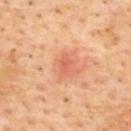Part of a total-body skin-imaging series; this lesion was reviewed on a skin check and was not flagged for biopsy.
Located on the upper back.
Automated image analysis of the tile measured a mean CIELAB color near L≈62 a*≈33 b*≈36, about 8 CIELAB-L* units darker than the surrounding skin, and a normalized lesion–skin contrast near 5. And it measured border irregularity of about 2.5 on a 0–10 scale, internal color variation of about 0 on a 0–10 scale, and radial color variation of about 0. It also reported a nevus-likeness score of about 0/100.
The subject is a male aged approximately 55.
Imaged with cross-polarized lighting.
Longest diameter approximately 2 mm.
A lesion tile, about 15 mm wide, cut from a 3D total-body photograph.